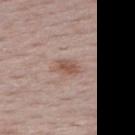{"patient": {"sex": "female", "age_approx": 50}, "image": {"source": "total-body photography crop", "field_of_view_mm": 15}, "site": "upper back"}